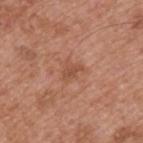lesion diameter = about 2.5 mm | body site = the upper back | TBP lesion metrics = a border-irregularity index near 3.5/10, a within-lesion color-variation index near 1.5/10, and peripheral color asymmetry of about 0.5; a nevus-likeness score of about 0/100 and a lesion-detection confidence of about 100/100 | lighting = white-light | patient = male, about 55 years old | image source = 15 mm crop, total-body photography.